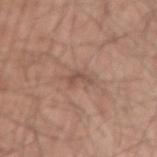This lesion was catalogued during total-body skin photography and was not selected for biopsy.
A male patient about 40 years old.
The lesion is on the left thigh.
A 15 mm close-up tile from a total-body photography series done for melanoma screening.
Measured at roughly 2.5 mm in maximum diameter.
Captured under white-light illumination.